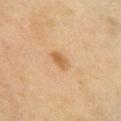- notes · total-body-photography surveillance lesion; no biopsy
- image-analysis metrics · a lesion color around L≈53 a*≈17 b*≈36 in CIELAB, about 8 CIELAB-L* units darker than the surrounding skin, and a normalized lesion–skin contrast near 6.5; a color-variation rating of about 3.5/10 and peripheral color asymmetry of about 1.5; an automated nevus-likeness rating near 75 out of 100 and a lesion-detection confidence of about 100/100
- patient · female, aged approximately 60
- tile lighting · cross-polarized illumination
- acquisition · ~15 mm tile from a whole-body skin photo
- body site · the front of the torso
- lesion size · ~2.5 mm (longest diameter)